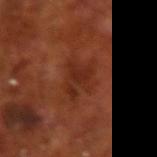Recorded during total-body skin imaging; not selected for excision or biopsy.
Automated tile analysis of the lesion measured an average lesion color of about L≈28 a*≈25 b*≈30 (CIELAB), about 6 CIELAB-L* units darker than the surrounding skin, and a lesion-to-skin contrast of about 6.5 (normalized; higher = more distinct). It also reported a nevus-likeness score of about 0/100.
The patient is a male aged 68–72.
Located on the right forearm.
Cropped from a total-body skin-imaging series; the visible field is about 15 mm.
The recorded lesion diameter is about 4.5 mm.